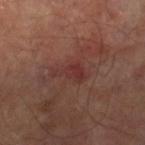Part of a total-body skin-imaging series; this lesion was reviewed on a skin check and was not flagged for biopsy.
A 15 mm close-up extracted from a 3D total-body photography capture.
The lesion is located on the right lower leg.
The tile uses cross-polarized illumination.
The recorded lesion diameter is about 4 mm.
The subject is a male roughly 65 years of age.